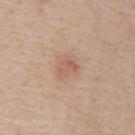Assessment: Part of a total-body skin-imaging series; this lesion was reviewed on a skin check and was not flagged for biopsy. Clinical summary: The patient is a male approximately 60 years of age. A 15 mm crop from a total-body photograph taken for skin-cancer surveillance. Located on the abdomen.